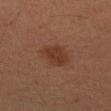| field | value |
|---|---|
| follow-up | no biopsy performed (imaged during a skin exam) |
| location | the left forearm |
| subject | female, aged 58–62 |
| imaging modality | ~15 mm crop, total-body skin-cancer survey |